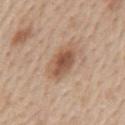A male subject about 60 years old. A 15 mm crop from a total-body photograph taken for skin-cancer surveillance. On the mid back.